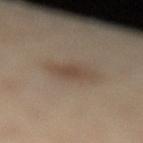biopsy status: no biopsy performed (imaged during a skin exam)
TBP lesion metrics: a lesion color around L≈46 a*≈13 b*≈25 in CIELAB, a lesion–skin lightness drop of about 8, and a lesion-to-skin contrast of about 6 (normalized; higher = more distinct)
imaging modality: ~15 mm crop, total-body skin-cancer survey
size: ~3 mm (longest diameter)
site: the leg
subject: female, aged approximately 45